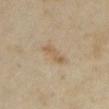Captured under cross-polarized illumination. The subject is a female aged approximately 35. About 3 mm across. Automated tile analysis of the lesion measured a footprint of about 3.5 mm² and two-axis asymmetry of about 0.4. And it measured a classifier nevus-likeness of about 20/100. A roughly 15 mm field-of-view crop from a total-body skin photograph. The lesion is on the front of the torso.